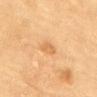This lesion was catalogued during total-body skin photography and was not selected for biopsy.
On the upper back.
Automated image analysis of the tile measured an area of roughly 3 mm², a shape eccentricity near 0.85, and two-axis asymmetry of about 0.3. And it measured border irregularity of about 2.5 on a 0–10 scale, a within-lesion color-variation index near 0.5/10, and peripheral color asymmetry of about 0. It also reported a classifier nevus-likeness of about 10/100 and lesion-presence confidence of about 100/100.
Captured under cross-polarized illumination.
Cropped from a whole-body photographic skin survey; the tile spans about 15 mm.
The lesion's longest dimension is about 2.5 mm.
A female patient, approximately 80 years of age.